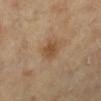Recorded during total-body skin imaging; not selected for excision or biopsy. From the left lower leg. The lesion's longest dimension is about 2.5 mm. This is a cross-polarized tile. A female patient approximately 60 years of age. A region of skin cropped from a whole-body photographic capture, roughly 15 mm wide.